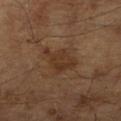| feature | finding |
|---|---|
| follow-up | total-body-photography surveillance lesion; no biopsy |
| TBP lesion metrics | a footprint of about 8 mm², an eccentricity of roughly 0.8, and a symmetry-axis asymmetry near 0.45; a mean CIELAB color near L≈32 a*≈17 b*≈28, a lesion–skin lightness drop of about 7, and a normalized lesion–skin contrast near 6.5; a border-irregularity index near 5.5/10 and a within-lesion color-variation index near 2/10; a nevus-likeness score of about 0/100 |
| patient | male, aged around 65 |
| imaging modality | ~15 mm tile from a whole-body skin photo |
| tile lighting | cross-polarized illumination |